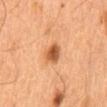Q: Was this lesion biopsied?
A: imaged on a skin check; not biopsied
Q: Patient demographics?
A: male, in their mid- to late 60s
Q: How was this image acquired?
A: total-body-photography crop, ~15 mm field of view
Q: Where on the body is the lesion?
A: the mid back